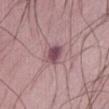Assessment:
Recorded during total-body skin imaging; not selected for excision or biopsy.
Clinical summary:
A roughly 15 mm field-of-view crop from a total-body skin photograph. The lesion is on the front of the torso. The subject is a male roughly 65 years of age.